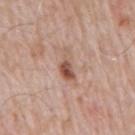Impression: Recorded during total-body skin imaging; not selected for excision or biopsy. Background: On the mid back. Measured at roughly 4.5 mm in maximum diameter. Captured under white-light illumination. A male subject aged approximately 50. A lesion tile, about 15 mm wide, cut from a 3D total-body photograph. An algorithmic analysis of the crop reported an area of roughly 5 mm² and two-axis asymmetry of about 0.4. It also reported a lesion color around L≈54 a*≈21 b*≈28 in CIELAB, a lesion–skin lightness drop of about 12, and a normalized border contrast of about 8.5. It also reported a border-irregularity index near 5/10, internal color variation of about 4.5 on a 0–10 scale, and peripheral color asymmetry of about 1. It also reported a classifier nevus-likeness of about 65/100 and a lesion-detection confidence of about 100/100.